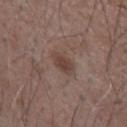<lesion>
<biopsy_status>not biopsied; imaged during a skin examination</biopsy_status>
<patient>
  <sex>male</sex>
  <age_approx>75</age_approx>
</patient>
<image>
  <source>total-body photography crop</source>
  <field_of_view_mm>15</field_of_view_mm>
</image>
<site>left forearm</site>
</lesion>